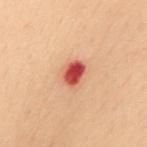diameter: ≈3 mm; patient: female, aged around 60; acquisition: ~15 mm crop, total-body skin-cancer survey; lighting: cross-polarized; site: the mid back.